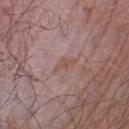Recorded during total-body skin imaging; not selected for excision or biopsy. The lesion's longest dimension is about 3 mm. A 15 mm crop from a total-body photograph taken for skin-cancer surveillance. From the right lower leg. Automated tile analysis of the lesion measured a lesion color around L≈51 a*≈19 b*≈24 in CIELAB, a lesion–skin lightness drop of about 6, and a normalized lesion–skin contrast near 6. It also reported a border-irregularity rating of about 5.5/10, internal color variation of about 0 on a 0–10 scale, and a peripheral color-asymmetry measure near 0. The software also gave a nevus-likeness score of about 0/100 and a detector confidence of about 95 out of 100 that the crop contains a lesion. A male patient roughly 65 years of age.